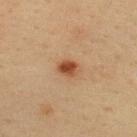| field | value |
|---|---|
| anatomic site | the back |
| lesion diameter | ≈2.5 mm |
| automated metrics | an average lesion color of about L≈43 a*≈23 b*≈33 (CIELAB) and roughly 12 lightness units darker than nearby skin; a classifier nevus-likeness of about 100/100 and a detector confidence of about 100 out of 100 that the crop contains a lesion |
| imaging modality | ~15 mm tile from a whole-body skin photo |
| subject | male, aged 38 to 42 |
| illumination | cross-polarized illumination |